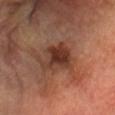No biopsy was performed on this lesion — it was imaged during a full skin examination and was not determined to be concerning. Measured at roughly 4 mm in maximum diameter. From the head or neck. Automated tile analysis of the lesion measured a symmetry-axis asymmetry near 0.3. It also reported a mean CIELAB color near L≈34 a*≈21 b*≈27. The software also gave a classifier nevus-likeness of about 60/100 and a detector confidence of about 100 out of 100 that the crop contains a lesion. A male patient, about 65 years old. A 15 mm close-up tile from a total-body photography series done for melanoma screening. The tile uses cross-polarized illumination.